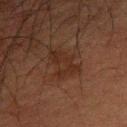Captured during whole-body skin photography for melanoma surveillance; the lesion was not biopsied. The lesion is on the left forearm. A lesion tile, about 15 mm wide, cut from a 3D total-body photograph. A male patient in their 60s.